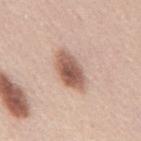acquisition: total-body-photography crop, ~15 mm field of view | lighting: white-light | subject: male, in their mid-40s | TBP lesion metrics: a mean CIELAB color near L≈57 a*≈20 b*≈27, a lesion–skin lightness drop of about 16, and a lesion-to-skin contrast of about 10 (normalized; higher = more distinct); border irregularity of about 2 on a 0–10 scale, a within-lesion color-variation index near 5/10, and radial color variation of about 1.5; lesion-presence confidence of about 100/100 | location: the mid back | lesion size: ≈5 mm.